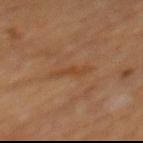biopsy status: total-body-photography surveillance lesion; no biopsy
automated metrics: a footprint of about 4.5 mm² and a shape-asymmetry score of about 0.3 (0 = symmetric); a lesion color around L≈40 a*≈19 b*≈32 in CIELAB, roughly 5 lightness units darker than nearby skin, and a normalized lesion–skin contrast near 5.5; border irregularity of about 4 on a 0–10 scale, a color-variation rating of about 0.5/10, and a peripheral color-asymmetry measure near 0
lesion diameter: ~4 mm (longest diameter)
site: the mid back
subject: male, aged 58–62
imaging modality: 15 mm crop, total-body photography